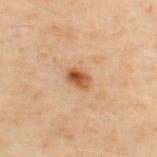Impression:
Recorded during total-body skin imaging; not selected for excision or biopsy.
Acquisition and patient details:
Located on the upper back. A male patient roughly 50 years of age. Captured under cross-polarized illumination. A region of skin cropped from a whole-body photographic capture, roughly 15 mm wide. The lesion-visualizer software estimated a footprint of about 4 mm² and two-axis asymmetry of about 0.25. The software also gave a mean CIELAB color near L≈45 a*≈21 b*≈33, a lesion–skin lightness drop of about 12, and a normalized lesion–skin contrast near 10.